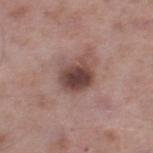Q: What did automated image analysis measure?
A: a lesion area of about 10 mm², a shape eccentricity near 0.55, and a symmetry-axis asymmetry near 0.25; a lesion color around L≈44 a*≈20 b*≈21 in CIELAB, about 14 CIELAB-L* units darker than the surrounding skin, and a normalized lesion–skin contrast near 10.5; border irregularity of about 2.5 on a 0–10 scale
Q: What is the imaging modality?
A: ~15 mm tile from a whole-body skin photo
Q: What are the patient's age and sex?
A: male, roughly 55 years of age
Q: What lighting was used for the tile?
A: white-light
Q: What is the anatomic site?
A: the right lower leg